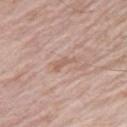biopsy status: no biopsy performed (imaged during a skin exam); site: the right thigh; patient: male, aged around 75; image: 15 mm crop, total-body photography.